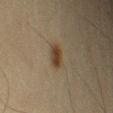biopsy status: imaged on a skin check; not biopsied | automated lesion analysis: a footprint of about 4.5 mm², a shape eccentricity near 0.7, and a symmetry-axis asymmetry near 0.15; a lesion color around L≈32 a*≈11 b*≈25 in CIELAB, roughly 8 lightness units darker than nearby skin, and a lesion-to-skin contrast of about 9 (normalized; higher = more distinct); a border-irregularity rating of about 2/10, a color-variation rating of about 3/10, and a peripheral color-asymmetry measure near 1 | location: the left upper arm | imaging modality: ~15 mm crop, total-body skin-cancer survey | patient: male, aged around 65.